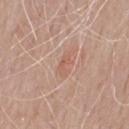The lesion was tiled from a total-body skin photograph and was not biopsied. A roughly 15 mm field-of-view crop from a total-body skin photograph. The recorded lesion diameter is about 2.5 mm. The lesion-visualizer software estimated a footprint of about 2.5 mm² and a symmetry-axis asymmetry near 0.35. And it measured a border-irregularity rating of about 3.5/10. The lesion is on the chest. Captured under white-light illumination. A male patient roughly 75 years of age.